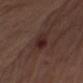  biopsy_status: not biopsied; imaged during a skin examination